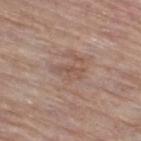Imaged during a routine full-body skin examination; the lesion was not biopsied and no histopathology is available. The lesion is on the leg. A region of skin cropped from a whole-body photographic capture, roughly 15 mm wide. The lesion's longest dimension is about 2.5 mm. A female patient, aged 68 to 72. The lesion-visualizer software estimated a lesion area of about 2 mm², an eccentricity of roughly 0.95, and two-axis asymmetry of about 0.45. And it measured a lesion color around L≈51 a*≈18 b*≈25 in CIELAB, a lesion–skin lightness drop of about 6, and a normalized lesion–skin contrast near 5. Captured under white-light illumination.